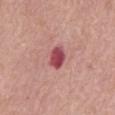biopsy status: catalogued during a skin exam; not biopsied | anatomic site: the abdomen | subject: female, about 65 years old | imaging modality: 15 mm crop, total-body photography.